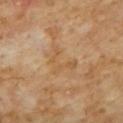follow-up = total-body-photography surveillance lesion; no biopsy
patient = male, roughly 60 years of age
anatomic site = the chest
acquisition = 15 mm crop, total-body photography